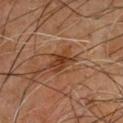Impression: Part of a total-body skin-imaging series; this lesion was reviewed on a skin check and was not flagged for biopsy. Acquisition and patient details: Located on the back. This image is a 15 mm lesion crop taken from a total-body photograph. The patient is a male aged approximately 60.